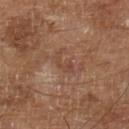notes = catalogued during a skin exam; not biopsied | patient = male, aged approximately 65 | acquisition = ~15 mm crop, total-body skin-cancer survey | illumination = cross-polarized illumination.